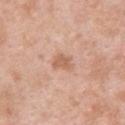biopsy_status: not biopsied; imaged during a skin examination
lesion_size:
  long_diameter_mm_approx: 2.5
automated_metrics:
  vs_skin_darker_L: 9.0
  vs_skin_contrast_norm: 6.5
  border_irregularity_0_10: 3.0
  color_variation_0_10: 1.5
  peripheral_color_asymmetry: 0.5
site: upper back
image:
  source: total-body photography crop
  field_of_view_mm: 15
patient:
  sex: male
  age_approx: 40
lighting: white-light